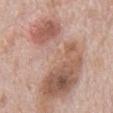Notes:
* workup · no biopsy performed (imaged during a skin exam)
* image · ~15 mm crop, total-body skin-cancer survey
* size · about 18 mm
* site · the abdomen
* subject · male, roughly 70 years of age
* tile lighting · white-light